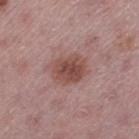The lesion is on the left thigh. The tile uses white-light illumination. The patient is a female aged around 45. A 15 mm crop from a total-body photograph taken for skin-cancer surveillance. The lesion-visualizer software estimated a border-irregularity index near 1.5/10, internal color variation of about 3.5 on a 0–10 scale, and radial color variation of about 1. The software also gave a nevus-likeness score of about 75/100.